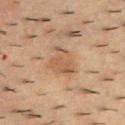Case summary:
– notes — imaged on a skin check; not biopsied
– subject — male, roughly 55 years of age
– acquisition — ~15 mm crop, total-body skin-cancer survey
– body site — the upper back
– automated metrics — an area of roughly 8.5 mm², a shape eccentricity near 0.6, and a shape-asymmetry score of about 0.4 (0 = symmetric); a within-lesion color-variation index near 3/10 and radial color variation of about 1; a nevus-likeness score of about 75/100
– tile lighting — cross-polarized